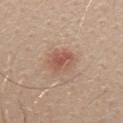follow-up: total-body-photography surveillance lesion; no biopsy
lesion diameter: ~3.5 mm (longest diameter)
subject: male, about 30 years old
illumination: white-light illumination
image: 15 mm crop, total-body photography
site: the mid back